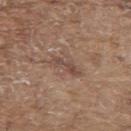Case summary:
– biopsy status: catalogued during a skin exam; not biopsied
– patient: male, aged around 80
– lesion diameter: about 4 mm
– illumination: white-light
– site: the upper back
– automated metrics: an automated nevus-likeness rating near 0 out of 100 and a lesion-detection confidence of about 90/100
– image source: ~15 mm crop, total-body skin-cancer survey